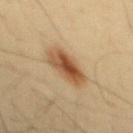notes = no biopsy performed (imaged during a skin exam); image = ~15 mm crop, total-body skin-cancer survey; anatomic site = the mid back; patient = male, aged approximately 45; tile lighting = cross-polarized.